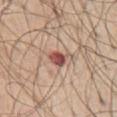Assessment: The lesion was photographed on a routine skin check and not biopsied; there is no pathology result. Acquisition and patient details: Imaged with white-light lighting. A male subject aged around 70. On the chest. The recorded lesion diameter is about 2.5 mm. A 15 mm close-up extracted from a 3D total-body photography capture.